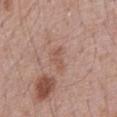| key | value |
|---|---|
| workup | imaged on a skin check; not biopsied |
| patient | male, about 70 years old |
| body site | the chest |
| lesion size | about 2.5 mm |
| tile lighting | white-light illumination |
| acquisition | total-body-photography crop, ~15 mm field of view |
| TBP lesion metrics | a lesion color around L≈54 a*≈21 b*≈27 in CIELAB, a lesion–skin lightness drop of about 7, and a lesion-to-skin contrast of about 5.5 (normalized; higher = more distinct); a border-irregularity rating of about 4.5/10 and peripheral color asymmetry of about 0 |